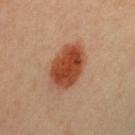lesion_size:
  long_diameter_mm_approx: 6.0
lighting: cross-polarized
patient:
  sex: female
  age_approx: 50
image:
  source: total-body photography crop
  field_of_view_mm: 15
automated_metrics:
  cielab_L: 46
  cielab_a: 28
  cielab_b: 35
  vs_skin_darker_L: 15.0
  vs_skin_contrast_norm: 11.0
  lesion_detection_confidence_0_100: 100